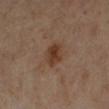Case summary:
- follow-up: imaged on a skin check; not biopsied
- acquisition: ~15 mm crop, total-body skin-cancer survey
- body site: the left lower leg
- automated lesion analysis: a classifier nevus-likeness of about 80/100
- tile lighting: cross-polarized illumination
- diameter: ≈3 mm
- patient: female, in their 70s